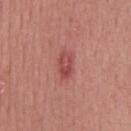This lesion was catalogued during total-body skin photography and was not selected for biopsy.
Longest diameter approximately 3.5 mm.
Located on the back.
A male patient aged 43–47.
The tile uses white-light illumination.
This image is a 15 mm lesion crop taken from a total-body photograph.